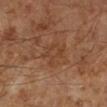follow-up=imaged on a skin check; not biopsied | patient=male, in their 60s | automated lesion analysis=a lesion area of about 5.5 mm², a shape eccentricity near 0.65, and a symmetry-axis asymmetry near 0.35 | location=the right lower leg | illumination=cross-polarized | size=≈3.5 mm | image=15 mm crop, total-body photography.